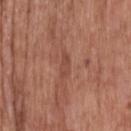biopsy status — imaged on a skin check; not biopsied
image source — ~15 mm tile from a whole-body skin photo
patient — male, in their 60s
tile lighting — white-light illumination
lesion size — ~3 mm (longest diameter)
anatomic site — the head or neck